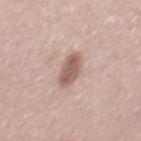Findings:
* notes · total-body-photography surveillance lesion; no biopsy